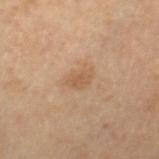The lesion was tiled from a total-body skin photograph and was not biopsied.
The recorded lesion diameter is about 3 mm.
The patient is a male aged 63 to 67.
The lesion is located on the right thigh.
Captured under cross-polarized illumination.
A region of skin cropped from a whole-body photographic capture, roughly 15 mm wide.
Automated image analysis of the tile measured a lesion area of about 4 mm². And it measured a lesion color around L≈57 a*≈19 b*≈34 in CIELAB, about 7 CIELAB-L* units darker than the surrounding skin, and a normalized border contrast of about 5.5. The analysis additionally found an automated nevus-likeness rating near 15 out of 100.